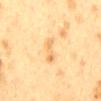No biopsy was performed on this lesion — it was imaged during a full skin examination and was not determined to be concerning. Approximately 4 mm at its widest. The lesion-visualizer software estimated an area of roughly 4 mm², an outline eccentricity of about 0.95 (0 = round, 1 = elongated), and a symmetry-axis asymmetry near 0.55. And it measured roughly 7 lightness units darker than nearby skin and a lesion-to-skin contrast of about 5 (normalized; higher = more distinct). The software also gave border irregularity of about 6 on a 0–10 scale, internal color variation of about 1.5 on a 0–10 scale, and peripheral color asymmetry of about 0. The lesion is located on the mid back. The patient is a female in their 40s. This is a cross-polarized tile. Cropped from a whole-body photographic skin survey; the tile spans about 15 mm.